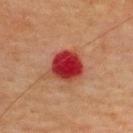acquisition: ~15 mm tile from a whole-body skin photo | lesion diameter: ~4.5 mm (longest diameter) | body site: the upper back | subject: female, aged 53 to 57 | automated lesion analysis: a shape eccentricity near 0.45; a normalized border contrast of about 12.5; a border-irregularity rating of about 2.5/10, a color-variation rating of about 5.5/10, and peripheral color asymmetry of about 2; a classifier nevus-likeness of about 0/100 and a detector confidence of about 100 out of 100 that the crop contains a lesion.